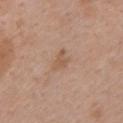Captured during whole-body skin photography for melanoma surveillance; the lesion was not biopsied. Approximately 3 mm at its widest. A roughly 15 mm field-of-view crop from a total-body skin photograph. A female subject, in their 40s. Automated tile analysis of the lesion measured an area of roughly 3.5 mm² and an eccentricity of roughly 0.75. The analysis additionally found a classifier nevus-likeness of about 0/100. Imaged with white-light lighting. The lesion is on the left upper arm.